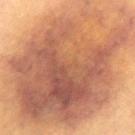Q: Was this lesion biopsied?
A: no biopsy performed (imaged during a skin exam)
Q: Patient demographics?
A: female, aged 78–82
Q: What kind of image is this?
A: ~15 mm tile from a whole-body skin photo
Q: What is the anatomic site?
A: the mid back
Q: How large is the lesion?
A: ≈18 mm
Q: How was the tile lit?
A: cross-polarized
Q: What did automated image analysis measure?
A: a lesion area of about 145 mm², an eccentricity of roughly 0.75, and a shape-asymmetry score of about 0.2 (0 = symmetric); a lesion color around L≈43 a*≈18 b*≈26 in CIELAB and a normalized lesion–skin contrast near 9.5; a detector confidence of about 90 out of 100 that the crop contains a lesion